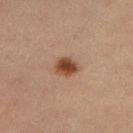This lesion was catalogued during total-body skin photography and was not selected for biopsy. A roughly 15 mm field-of-view crop from a total-body skin photograph. The lesion is on the left thigh. The patient is a female aged 28–32.